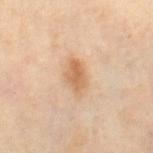  biopsy_status: not biopsied; imaged during a skin examination
  site: right thigh
  image:
    source: total-body photography crop
    field_of_view_mm: 15
  lesion_size:
    long_diameter_mm_approx: 4.0
  patient:
    sex: female
    age_approx: 50
  lighting: cross-polarized